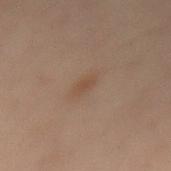This lesion was catalogued during total-body skin photography and was not selected for biopsy. On the mid back. The subject is a female in their mid- to late 50s. Imaged with cross-polarized lighting. This image is a 15 mm lesion crop taken from a total-body photograph.